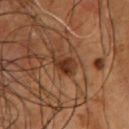biopsy status: imaged on a skin check; not biopsied | image: ~15 mm crop, total-body skin-cancer survey | lighting: cross-polarized illumination | subject: male, roughly 55 years of age | location: the chest | automated lesion analysis: a mean CIELAB color near L≈31 a*≈20 b*≈29 and about 10 CIELAB-L* units darker than the surrounding skin; a border-irregularity index near 2.5/10, a color-variation rating of about 4/10, and peripheral color asymmetry of about 1.5 | size: ~3.5 mm (longest diameter).